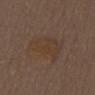{"patient": {"sex": "male", "age_approx": 70}, "lesion_size": {"long_diameter_mm_approx": 5.0}, "site": "chest", "lighting": "white-light", "image": {"source": "total-body photography crop", "field_of_view_mm": 15}, "automated_metrics": {"area_mm2_approx": 18.0, "eccentricity": 0.55, "shape_asymmetry": 0.35}}